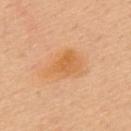Part of a total-body skin-imaging series; this lesion was reviewed on a skin check and was not flagged for biopsy.
A male subject aged around 50.
About 5 mm across.
This image is a 15 mm lesion crop taken from a total-body photograph.
From the mid back.